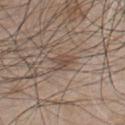Notes:
- body site · the chest
- size · ≈3 mm
- patient · male, roughly 50 years of age
- imaging modality · ~15 mm crop, total-body skin-cancer survey